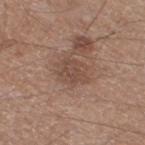Assessment: This lesion was catalogued during total-body skin photography and was not selected for biopsy. Background: Longest diameter approximately 4 mm. Located on the right thigh. Captured under white-light illumination. This image is a 15 mm lesion crop taken from a total-body photograph. The subject is a male aged 63 to 67. The total-body-photography lesion software estimated a lesion area of about 8 mm², an outline eccentricity of about 0.65 (0 = round, 1 = elongated), and two-axis asymmetry of about 0.2. It also reported internal color variation of about 2 on a 0–10 scale and radial color variation of about 1.